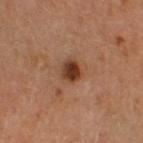site: right thigh
automated_metrics:
  cielab_L: 38
  cielab_a: 23
  cielab_b: 32
  vs_skin_darker_L: 14.0
  vs_skin_contrast_norm: 11.5
  border_irregularity_0_10: 2.0
  color_variation_0_10: 5.5
  peripheral_color_asymmetry: 2.0
patient:
  sex: male
  age_approx: 65
image:
  source: total-body photography crop
  field_of_view_mm: 15